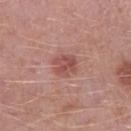biopsy status: total-body-photography surveillance lesion; no biopsy
anatomic site: the right lower leg
patient: male, aged 48 to 52
illumination: white-light illumination
automated metrics: an eccentricity of roughly 0.45 and a shape-asymmetry score of about 0.2 (0 = symmetric); an automated nevus-likeness rating near 75 out of 100 and lesion-presence confidence of about 100/100
lesion diameter: ~3 mm (longest diameter)
imaging modality: total-body-photography crop, ~15 mm field of view